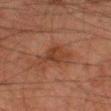biopsy status: no biopsy performed (imaged during a skin exam) | imaging modality: ~15 mm crop, total-body skin-cancer survey | patient: male, aged approximately 80 | site: the left thigh | TBP lesion metrics: an area of roughly 5.5 mm² and two-axis asymmetry of about 0.35; a lesion color around L≈30 a*≈19 b*≈26 in CIELAB, a lesion–skin lightness drop of about 7, and a normalized lesion–skin contrast near 7 | illumination: cross-polarized illumination.